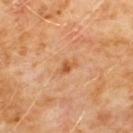Impression: Captured during whole-body skin photography for melanoma surveillance; the lesion was not biopsied. Image and clinical context: The subject is a male aged approximately 60. An algorithmic analysis of the crop reported a detector confidence of about 100 out of 100 that the crop contains a lesion. A close-up tile cropped from a whole-body skin photograph, about 15 mm across. Measured at roughly 2.5 mm in maximum diameter. The lesion is located on the chest. Imaged with cross-polarized lighting.